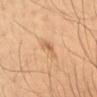Image and clinical context:
A 15 mm close-up extracted from a 3D total-body photography capture. The recorded lesion diameter is about 2.5 mm. Automated image analysis of the tile measured an average lesion color of about L≈56 a*≈19 b*≈35 (CIELAB). And it measured an automated nevus-likeness rating near 30 out of 100 and a detector confidence of about 100 out of 100 that the crop contains a lesion. Located on the lower back. The patient is a male approximately 35 years of age. The tile uses cross-polarized illumination.